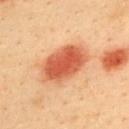biopsy_status: not biopsied; imaged during a skin examination
lighting: cross-polarized
site: back
lesion_size:
  long_diameter_mm_approx: 6.5
image:
  source: total-body photography crop
  field_of_view_mm: 15
patient:
  sex: male
  age_approx: 40